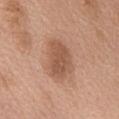Assessment:
No biopsy was performed on this lesion — it was imaged during a full skin examination and was not determined to be concerning.
Image and clinical context:
Approximately 5 mm at its widest. A female patient, aged approximately 60. Imaged with white-light lighting. A 15 mm close-up extracted from a 3D total-body photography capture. The lesion is on the abdomen.